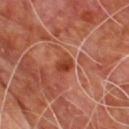Recorded during total-body skin imaging; not selected for excision or biopsy.
A 15 mm crop from a total-body photograph taken for skin-cancer surveillance.
The lesion is located on the upper back.
Automated image analysis of the tile measured a symmetry-axis asymmetry near 0.3. And it measured a lesion color around L≈41 a*≈30 b*≈34 in CIELAB, roughly 10 lightness units darker than nearby skin, and a normalized border contrast of about 8. The software also gave a border-irregularity index near 2.5/10 and radial color variation of about 1.5.
Longest diameter approximately 2.5 mm.
Imaged with cross-polarized lighting.
The subject is a male about 60 years old.